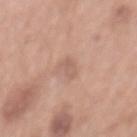follow-up = no biopsy performed (imaged during a skin exam) | acquisition = ~15 mm tile from a whole-body skin photo | patient = male, roughly 70 years of age | image-analysis metrics = a lesion color around L≈60 a*≈19 b*≈27 in CIELAB and a lesion–skin lightness drop of about 7; border irregularity of about 3.5 on a 0–10 scale, internal color variation of about 1 on a 0–10 scale, and radial color variation of about 0.5 | location = the mid back | size = ~3 mm (longest diameter) | tile lighting = white-light.